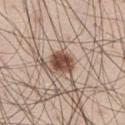biopsy_status: not biopsied; imaged during a skin examination
lesion_size:
  long_diameter_mm_approx: 3.0
automated_metrics:
  area_mm2_approx: 6.0
  eccentricity: 0.65
  nevus_likeness_0_100: 100
  lesion_detection_confidence_0_100: 100
patient:
  sex: male
  age_approx: 30
site: left thigh
image:
  source: total-body photography crop
  field_of_view_mm: 15
lighting: white-light